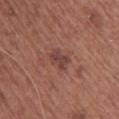Captured during whole-body skin photography for melanoma surveillance; the lesion was not biopsied.
Cropped from a total-body skin-imaging series; the visible field is about 15 mm.
Measured at roughly 2.5 mm in maximum diameter.
The lesion is on the upper back.
Captured under white-light illumination.
The subject is a female roughly 60 years of age.
Automated image analysis of the tile measured a nevus-likeness score of about 5/100.